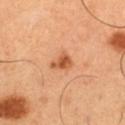Q: Was a biopsy performed?
A: imaged on a skin check; not biopsied
Q: How was the tile lit?
A: cross-polarized illumination
Q: What is the imaging modality?
A: 15 mm crop, total-body photography
Q: Lesion size?
A: about 2.5 mm
Q: What is the anatomic site?
A: the left thigh
Q: What are the patient's age and sex?
A: male, aged around 50